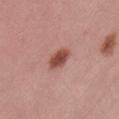Q: Was a biopsy performed?
A: total-body-photography surveillance lesion; no biopsy
Q: Where on the body is the lesion?
A: the lower back
Q: What kind of image is this?
A: 15 mm crop, total-body photography
Q: Automated lesion metrics?
A: an outline eccentricity of about 0.65 (0 = round, 1 = elongated); a detector confidence of about 100 out of 100 that the crop contains a lesion
Q: How large is the lesion?
A: ~3 mm (longest diameter)
Q: How was the tile lit?
A: white-light illumination
Q: Who is the patient?
A: female, roughly 55 years of age